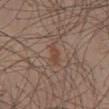<tbp_lesion>
<biopsy_status>not biopsied; imaged during a skin examination</biopsy_status>
<site>lower back</site>
<automated_metrics>
  <area_mm2_approx>3.5</area_mm2_approx>
  <eccentricity>0.9</eccentricity>
  <shape_asymmetry>0.35</shape_asymmetry>
  <border_irregularity_0_10>4.0</border_irregularity_0_10>
  <peripheral_color_asymmetry>0.5</peripheral_color_asymmetry>
</automated_metrics>
<image>
  <source>total-body photography crop</source>
  <field_of_view_mm>15</field_of_view_mm>
</image>
<lighting>white-light</lighting>
<patient>
  <sex>male</sex>
  <age_approx>35</age_approx>
</patient>
<lesion_size>
  <long_diameter_mm_approx>3.0</long_diameter_mm_approx>
</lesion_size>
</tbp_lesion>